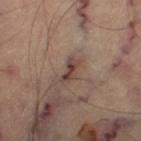Clinical impression:
Captured during whole-body skin photography for melanoma surveillance; the lesion was not biopsied.
Acquisition and patient details:
The lesion's longest dimension is about 3 mm. From the right thigh. A 15 mm close-up extracted from a 3D total-body photography capture. Imaged with cross-polarized lighting. Automated image analysis of the tile measured a mean CIELAB color near L≈42 a*≈18 b*≈21 and roughly 11 lightness units darker than nearby skin. The analysis additionally found border irregularity of about 5 on a 0–10 scale and internal color variation of about 0 on a 0–10 scale. And it measured a classifier nevus-likeness of about 0/100.